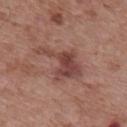Image and clinical context:
A male subject, in their mid- to late 50s. A roughly 15 mm field-of-view crop from a total-body skin photograph. Measured at roughly 6 mm in maximum diameter. On the mid back. The tile uses white-light illumination. Automated tile analysis of the lesion measured border irregularity of about 7.5 on a 0–10 scale, a color-variation rating of about 3.5/10, and peripheral color asymmetry of about 1. The software also gave a lesion-detection confidence of about 100/100.